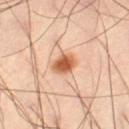Imaged during a routine full-body skin examination; the lesion was not biopsied and no histopathology is available.
Captured under cross-polarized illumination.
A 15 mm crop from a total-body photograph taken for skin-cancer surveillance.
The subject is a male aged 38 to 42.
About 3 mm across.
An algorithmic analysis of the crop reported an area of roughly 6 mm², an eccentricity of roughly 0.55, and two-axis asymmetry of about 0.1. The analysis additionally found a lesion color around L≈59 a*≈26 b*≈38 in CIELAB, roughly 15 lightness units darker than nearby skin, and a normalized lesion–skin contrast near 10.5. And it measured border irregularity of about 1 on a 0–10 scale, internal color variation of about 3.5 on a 0–10 scale, and radial color variation of about 1. The software also gave a classifier nevus-likeness of about 100/100.
The lesion is located on the leg.